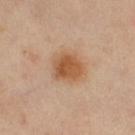Impression: Part of a total-body skin-imaging series; this lesion was reviewed on a skin check and was not flagged for biopsy. Clinical summary: A 15 mm crop from a total-body photograph taken for skin-cancer surveillance. Imaged with cross-polarized lighting. The lesion-visualizer software estimated an automated nevus-likeness rating near 100 out of 100 and lesion-presence confidence of about 100/100. The lesion is located on the right thigh. The subject is a female aged 43 to 47.